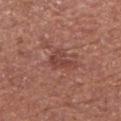biopsy_status: not biopsied; imaged during a skin examination
patient:
  sex: male
  age_approx: 55
automated_metrics:
  area_mm2_approx: 5.0
  eccentricity: 0.8
  shape_asymmetry: 0.45
  cielab_L: 43
  cielab_a: 25
  cielab_b: 25
  vs_skin_darker_L: 8.0
  vs_skin_contrast_norm: 6.5
  nevus_likeness_0_100: 0
  lesion_detection_confidence_0_100: 100
site: arm
lighting: white-light
image:
  source: total-body photography crop
  field_of_view_mm: 15